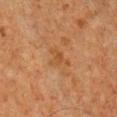Captured during whole-body skin photography for melanoma surveillance; the lesion was not biopsied. The lesion is located on the chest. A 15 mm crop from a total-body photograph taken for skin-cancer surveillance. The tile uses cross-polarized illumination. The lesion-visualizer software estimated a lesion area of about 3.5 mm², a shape eccentricity near 0.65, and a symmetry-axis asymmetry near 0.55. And it measured a border-irregularity rating of about 5.5/10, a color-variation rating of about 2/10, and radial color variation of about 1. The software also gave a classifier nevus-likeness of about 0/100. A male subject, approximately 65 years of age. About 3 mm across.